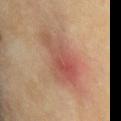Findings:
- image source: ~15 mm tile from a whole-body skin photo
- lesion diameter: about 6 mm
- site: the chest
- tile lighting: cross-polarized
- patient: female, in their 50s